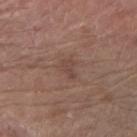workup — catalogued during a skin exam; not biopsied | TBP lesion metrics — an outline eccentricity of about 0.75 (0 = round, 1 = elongated) and a symmetry-axis asymmetry near 0.4; a mean CIELAB color near L≈44 a*≈17 b*≈23, about 6 CIELAB-L* units darker than the surrounding skin, and a normalized lesion–skin contrast near 5 | lighting — white-light illumination | image — ~15 mm tile from a whole-body skin photo | lesion size — about 2.5 mm | location — the arm | subject — male, aged 63–67.